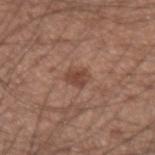Q: How large is the lesion?
A: ~2.5 mm (longest diameter)
Q: What are the patient's age and sex?
A: male, aged around 35
Q: Lesion location?
A: the left forearm
Q: How was the tile lit?
A: white-light
Q: What kind of image is this?
A: total-body-photography crop, ~15 mm field of view